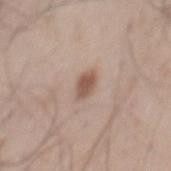{"biopsy_status": "not biopsied; imaged during a skin examination", "patient": {"sex": "male", "age_approx": 50}, "lesion_size": {"long_diameter_mm_approx": 3.0}, "site": "mid back", "image": {"source": "total-body photography crop", "field_of_view_mm": 15}, "lighting": "white-light", "automated_metrics": {"area_mm2_approx": 4.5, "eccentricity": 0.85, "shape_asymmetry": 0.15, "vs_skin_darker_L": 12.0, "vs_skin_contrast_norm": 8.0, "color_variation_0_10": 2.5, "nevus_likeness_0_100": 90}}